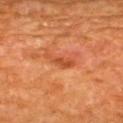This lesion was catalogued during total-body skin photography and was not selected for biopsy. A 15 mm close-up extracted from a 3D total-body photography capture. Located on the upper back. The lesion's longest dimension is about 4 mm. Imaged with cross-polarized lighting. A male subject aged 58 to 62. The total-body-photography lesion software estimated a footprint of about 4.5 mm², an eccentricity of roughly 0.9, and two-axis asymmetry of about 0.4. The analysis additionally found an average lesion color of about L≈48 a*≈31 b*≈40 (CIELAB). It also reported a classifier nevus-likeness of about 0/100 and a lesion-detection confidence of about 100/100.